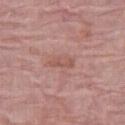No biopsy was performed on this lesion — it was imaged during a full skin examination and was not determined to be concerning. A female subject in their mid-60s. A 15 mm crop from a total-body photograph taken for skin-cancer surveillance. The lesion is on the leg.